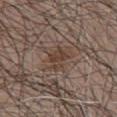Assessment: Imaged during a routine full-body skin examination; the lesion was not biopsied and no histopathology is available. Acquisition and patient details: The total-body-photography lesion software estimated a lesion color around L≈40 a*≈15 b*≈23 in CIELAB and a lesion-to-skin contrast of about 6.5 (normalized; higher = more distinct). The software also gave a nevus-likeness score of about 5/100 and lesion-presence confidence of about 95/100. Imaged with white-light lighting. A 15 mm crop from a total-body photograph taken for skin-cancer surveillance. Approximately 3 mm at its widest. On the chest. A male subject, aged approximately 65.